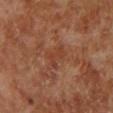image source = ~15 mm tile from a whole-body skin photo | patient = male, aged approximately 70 | site = the left lower leg.